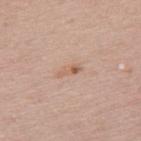This lesion was catalogued during total-body skin photography and was not selected for biopsy. This is a white-light tile. The patient is a female about 50 years old. A 15 mm close-up tile from a total-body photography series done for melanoma screening. Located on the back. The recorded lesion diameter is about 3 mm.